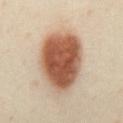Case summary:
* biopsy status · imaged on a skin check; not biopsied
* lesion size · about 7.5 mm
* patient · female, aged 38 to 42
* TBP lesion metrics · a lesion–skin lightness drop of about 20 and a lesion-to-skin contrast of about 12.5 (normalized; higher = more distinct); a nevus-likeness score of about 100/100
* location · the front of the torso
* imaging modality · ~15 mm tile from a whole-body skin photo
* lighting · cross-polarized